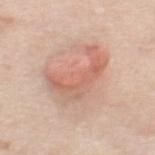Impression: Part of a total-body skin-imaging series; this lesion was reviewed on a skin check and was not flagged for biopsy. Clinical summary: The tile uses white-light illumination. On the mid back. About 6.5 mm across. The patient is a female aged 38 to 42. An algorithmic analysis of the crop reported an area of roughly 17 mm², an eccentricity of roughly 0.85, and a symmetry-axis asymmetry near 0.55. A roughly 15 mm field-of-view crop from a total-body skin photograph.